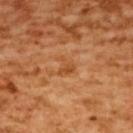Assessment:
No biopsy was performed on this lesion — it was imaged during a full skin examination and was not determined to be concerning.
Acquisition and patient details:
Measured at roughly 2.5 mm in maximum diameter. Located on the upper back. A female subject, aged 53 to 57. A 15 mm close-up extracted from a 3D total-body photography capture. Captured under cross-polarized illumination.